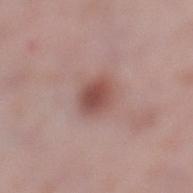Recorded during total-body skin imaging; not selected for excision or biopsy.